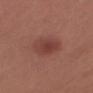<record>
  <biopsy_status>not biopsied; imaged during a skin examination</biopsy_status>
</record>